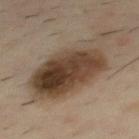biopsy_status: not biopsied; imaged during a skin examination
automated_metrics:
  cielab_L: 40
  cielab_a: 13
  cielab_b: 26
  vs_skin_darker_L: 14.0
  vs_skin_contrast_norm: 11.5
image:
  source: total-body photography crop
  field_of_view_mm: 15
site: back
lesion_size:
  long_diameter_mm_approx: 9.5
patient:
  sex: male
  age_approx: 40
lighting: cross-polarized